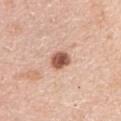No biopsy was performed on this lesion — it was imaged during a full skin examination and was not determined to be concerning.